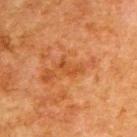Clinical impression: This lesion was catalogued during total-body skin photography and was not selected for biopsy. Clinical summary: The lesion is on the upper back. A 15 mm close-up extracted from a 3D total-body photography capture. The lesion's longest dimension is about 3 mm. The tile uses cross-polarized illumination. The subject is a male aged 78–82.